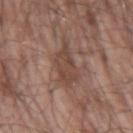Impression: The lesion was photographed on a routine skin check and not biopsied; there is no pathology result. Image and clinical context: This is a white-light tile. On the right upper arm. This image is a 15 mm lesion crop taken from a total-body photograph. A male patient, aged around 65. Measured at roughly 5.5 mm in maximum diameter.